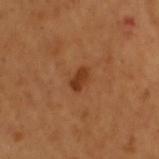Q: Is there a histopathology result?
A: no biopsy performed (imaged during a skin exam)